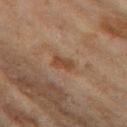The lesion was tiled from a total-body skin photograph and was not biopsied. A female patient, aged approximately 60. The lesion is on the left thigh. The tile uses cross-polarized illumination. Cropped from a total-body skin-imaging series; the visible field is about 15 mm. The recorded lesion diameter is about 2.5 mm.